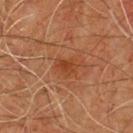Impression:
Imaged during a routine full-body skin examination; the lesion was not biopsied and no histopathology is available.